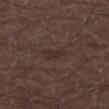Q: Patient demographics?
A: male, roughly 30 years of age
Q: How was this image acquired?
A: ~15 mm crop, total-body skin-cancer survey
Q: What lighting was used for the tile?
A: white-light illumination
Q: How large is the lesion?
A: ≈3 mm
Q: Lesion location?
A: the left lower leg